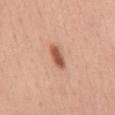biopsy status: no biopsy performed (imaged during a skin exam)
subject: female, aged approximately 35
image source: ~15 mm crop, total-body skin-cancer survey
site: the mid back